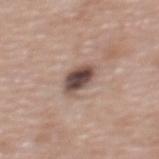| field | value |
|---|---|
| location | the upper back |
| subject | female, aged approximately 60 |
| image source | 15 mm crop, total-body photography |
| diameter | ~3.5 mm (longest diameter) |
| illumination | white-light illumination |